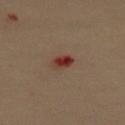follow-up: catalogued during a skin exam; not biopsied | diameter: ≈2.5 mm | site: the upper back | patient: female, about 60 years old | tile lighting: cross-polarized illumination | image-analysis metrics: a lesion color around L≈30 a*≈22 b*≈23 in CIELAB, about 10 CIELAB-L* units darker than the surrounding skin, and a lesion-to-skin contrast of about 10 (normalized; higher = more distinct); a lesion-detection confidence of about 100/100 | imaging modality: 15 mm crop, total-body photography.